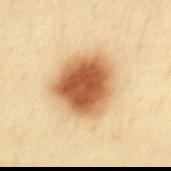Clinical impression:
Part of a total-body skin-imaging series; this lesion was reviewed on a skin check and was not flagged for biopsy.
Context:
A close-up tile cropped from a whole-body skin photograph, about 15 mm across. From the mid back. Imaged with cross-polarized lighting. Automated tile analysis of the lesion measured a mean CIELAB color near L≈53 a*≈20 b*≈37, about 18 CIELAB-L* units darker than the surrounding skin, and a normalized lesion–skin contrast near 12. The software also gave a nevus-likeness score of about 100/100 and lesion-presence confidence of about 100/100. Longest diameter approximately 6 mm. A male subject, roughly 35 years of age.